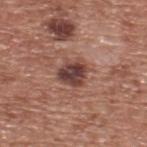Findings:
* workup: no biopsy performed (imaged during a skin exam)
* imaging modality: total-body-photography crop, ~15 mm field of view
* lesion diameter: about 3.5 mm
* illumination: white-light
* subject: male, roughly 75 years of age
* location: the upper back